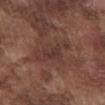Impression: No biopsy was performed on this lesion — it was imaged during a full skin examination and was not determined to be concerning. Context: A 15 mm close-up extracted from a 3D total-body photography capture. The lesion is located on the front of the torso. Automated tile analysis of the lesion measured an outline eccentricity of about 0.9 (0 = round, 1 = elongated). And it measured a color-variation rating of about 3.5/10. The analysis additionally found a classifier nevus-likeness of about 0/100 and lesion-presence confidence of about 85/100. This is a white-light tile. The recorded lesion diameter is about 5.5 mm. A male subject aged around 75.